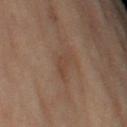No biopsy was performed on this lesion — it was imaged during a full skin examination and was not determined to be concerning.
A male patient, roughly 70 years of age.
A 15 mm close-up tile from a total-body photography series done for melanoma screening.
The lesion is located on the left upper arm.